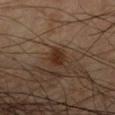Impression: No biopsy was performed on this lesion — it was imaged during a full skin examination and was not determined to be concerning. Image and clinical context: This is a cross-polarized tile. This image is a 15 mm lesion crop taken from a total-body photograph. The lesion is located on the right upper arm. A male subject approximately 65 years of age.